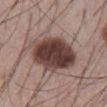Recorded during total-body skin imaging; not selected for excision or biopsy.
A lesion tile, about 15 mm wide, cut from a 3D total-body photograph.
A male subject, approximately 55 years of age.
The lesion is on the abdomen.